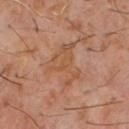Imaged with cross-polarized lighting. A roughly 15 mm field-of-view crop from a total-body skin photograph. A male patient aged 58 to 62. The lesion is on the chest. Automated tile analysis of the lesion measured a footprint of about 12 mm², a shape eccentricity near 0.65, and a symmetry-axis asymmetry near 0.6. The software also gave border irregularity of about 8 on a 0–10 scale and a peripheral color-asymmetry measure near 1. And it measured an automated nevus-likeness rating near 0 out of 100 and a lesion-detection confidence of about 95/100.